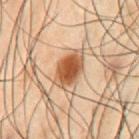Captured during whole-body skin photography for melanoma surveillance; the lesion was not biopsied.